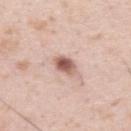Q: Is there a histopathology result?
A: total-body-photography surveillance lesion; no biopsy
Q: Where on the body is the lesion?
A: the upper back
Q: How was the tile lit?
A: white-light
Q: What are the patient's age and sex?
A: male, in their mid- to late 30s
Q: What is the imaging modality?
A: 15 mm crop, total-body photography
Q: How large is the lesion?
A: ≈2.5 mm